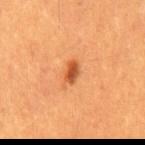<tbp_lesion>
<biopsy_status>not biopsied; imaged during a skin examination</biopsy_status>
<lesion_size>
  <long_diameter_mm_approx>2.5</long_diameter_mm_approx>
</lesion_size>
<image>
  <source>total-body photography crop</source>
  <field_of_view_mm>15</field_of_view_mm>
</image>
<site>back</site>
<automated_metrics>
  <cielab_L>50</cielab_L>
  <cielab_a>30</cielab_a>
  <cielab_b>42</cielab_b>
  <vs_skin_darker_L>13.0</vs_skin_darker_L>
  <vs_skin_contrast_norm>9.0</vs_skin_contrast_norm>
  <nevus_likeness_0_100>100</nevus_likeness_0_100>
  <lesion_detection_confidence_0_100>100</lesion_detection_confidence_0_100>
</automated_metrics>
<patient>
  <sex>male</sex>
  <age_approx>60</age_approx>
</patient>
</tbp_lesion>